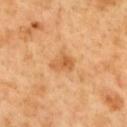Captured during whole-body skin photography for melanoma surveillance; the lesion was not biopsied. The lesion is on the back. Approximately 3 mm at its widest. A 15 mm close-up tile from a total-body photography series done for melanoma screening. A male subject, roughly 50 years of age. The tile uses cross-polarized illumination. Automated image analysis of the tile measured an eccentricity of roughly 0.75 and a shape-asymmetry score of about 0.4 (0 = symmetric). And it measured an average lesion color of about L≈60 a*≈25 b*≈43 (CIELAB), roughly 9 lightness units darker than nearby skin, and a normalized lesion–skin contrast near 6.5.